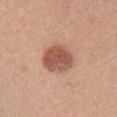This lesion was catalogued during total-body skin photography and was not selected for biopsy. Longest diameter approximately 4.5 mm. Cropped from a whole-body photographic skin survey; the tile spans about 15 mm. The lesion is on the chest. A female patient, in their mid- to late 20s. The lesion-visualizer software estimated a footprint of about 12 mm², an eccentricity of roughly 0.65, and two-axis asymmetry of about 0.15. It also reported border irregularity of about 1.5 on a 0–10 scale, internal color variation of about 4 on a 0–10 scale, and radial color variation of about 1. The analysis additionally found an automated nevus-likeness rating near 100 out of 100 and lesion-presence confidence of about 100/100. Imaged with white-light lighting.